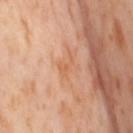Context: An algorithmic analysis of the crop reported a lesion-detection confidence of about 100/100. This image is a 15 mm lesion crop taken from a total-body photograph. A female patient aged 53–57. Captured under cross-polarized illumination. From the right thigh.